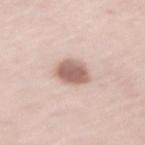Assessment:
This lesion was catalogued during total-body skin photography and was not selected for biopsy.
Acquisition and patient details:
The tile uses white-light illumination. A 15 mm close-up tile from a total-body photography series done for melanoma screening. A female patient, aged 63 to 67. Automated tile analysis of the lesion measured an outline eccentricity of about 0.45 (0 = round, 1 = elongated) and a symmetry-axis asymmetry near 0.15. The analysis additionally found a lesion-to-skin contrast of about 9.5 (normalized; higher = more distinct). And it measured a color-variation rating of about 3.5/10 and radial color variation of about 1. On the left upper arm. The lesion's longest dimension is about 3.5 mm.